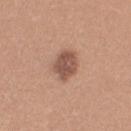Image and clinical context: The lesion's longest dimension is about 4 mm. The lesion is located on the arm. Captured under white-light illumination. A female subject, about 20 years old. This image is a 15 mm lesion crop taken from a total-body photograph.